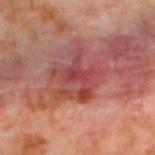<case>
<biopsy_status>not biopsied; imaged during a skin examination</biopsy_status>
</case>